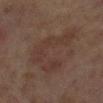Assessment:
Recorded during total-body skin imaging; not selected for excision or biopsy.
Background:
The lesion is on the right lower leg. A region of skin cropped from a whole-body photographic capture, roughly 15 mm wide. This is a cross-polarized tile. The patient is a female about 60 years old. The lesion-visualizer software estimated a lesion area of about 21 mm², an eccentricity of roughly 0.6, and two-axis asymmetry of about 0.6.